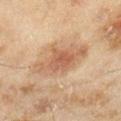The lesion was photographed on a routine skin check and not biopsied; there is no pathology result.
A female subject, aged around 60.
This is a cross-polarized tile.
Automated image analysis of the tile measured an area of roughly 17 mm², an outline eccentricity of about 0.85 (0 = round, 1 = elongated), and a symmetry-axis asymmetry near 0.3.
The lesion is on the right lower leg.
A close-up tile cropped from a whole-body skin photograph, about 15 mm across.